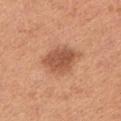- notes · total-body-photography surveillance lesion; no biopsy
- anatomic site · the left upper arm
- illumination · white-light illumination
- imaging modality · total-body-photography crop, ~15 mm field of view
- diameter · ~4.5 mm (longest diameter)
- subject · male, aged approximately 65